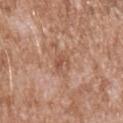workup: imaged on a skin check; not biopsied
patient: male, about 45 years old
site: the left upper arm
image: 15 mm crop, total-body photography
tile lighting: white-light
size: about 3 mm